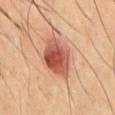Clinical impression:
This lesion was catalogued during total-body skin photography and was not selected for biopsy.
Background:
The lesion-visualizer software estimated a mean CIELAB color near L≈50 a*≈27 b*≈30, about 15 CIELAB-L* units darker than the surrounding skin, and a lesion-to-skin contrast of about 10 (normalized; higher = more distinct). It also reported lesion-presence confidence of about 100/100. On the chest. Imaged with cross-polarized lighting. A male subject aged 58 to 62. A close-up tile cropped from a whole-body skin photograph, about 15 mm across. Longest diameter approximately 5 mm.